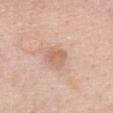<record>
<biopsy_status>not biopsied; imaged during a skin examination</biopsy_status>
<site>chest</site>
<lighting>white-light</lighting>
<image>
  <source>total-body photography crop</source>
  <field_of_view_mm>15</field_of_view_mm>
</image>
<patient>
  <sex>female</sex>
  <age_approx>60</age_approx>
</patient>
<lesion_size>
  <long_diameter_mm_approx>3.0</long_diameter_mm_approx>
</lesion_size>
</record>